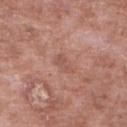Impression: The lesion was photographed on a routine skin check and not biopsied; there is no pathology result. Background: The patient is a male aged 53–57. The recorded lesion diameter is about 2.5 mm. Captured under white-light illumination. Located on the right lower leg. Cropped from a whole-body photographic skin survey; the tile spans about 15 mm.